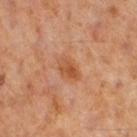Assessment:
No biopsy was performed on this lesion — it was imaged during a full skin examination and was not determined to be concerning.
Clinical summary:
Captured under cross-polarized illumination. The lesion is located on the right thigh. A male subject about 60 years old. Longest diameter approximately 3.5 mm. This image is a 15 mm lesion crop taken from a total-body photograph.